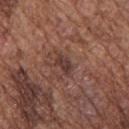workup: no biopsy performed (imaged during a skin exam)
automated lesion analysis: a border-irregularity rating of about 3.5/10 and a within-lesion color-variation index near 2.5/10
lighting: white-light illumination
body site: the upper back
patient: male, aged 73–77
image source: ~15 mm tile from a whole-body skin photo